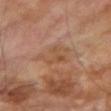The total-body-photography lesion software estimated a border-irregularity index near 5.5/10. And it measured a detector confidence of about 100 out of 100 that the crop contains a lesion. A male subject approximately 70 years of age. A roughly 15 mm field-of-view crop from a total-body skin photograph. Imaged with cross-polarized lighting. The lesion is located on the right upper arm. Measured at roughly 3.5 mm in maximum diameter.